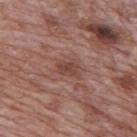Part of a total-body skin-imaging series; this lesion was reviewed on a skin check and was not flagged for biopsy. A male patient, aged approximately 70. Longest diameter approximately 2.5 mm. Automated tile analysis of the lesion measured a lesion color around L≈44 a*≈21 b*≈24 in CIELAB, roughly 8 lightness units darker than nearby skin, and a lesion-to-skin contrast of about 6.5 (normalized; higher = more distinct). It also reported border irregularity of about 2 on a 0–10 scale and a peripheral color-asymmetry measure near 0.5. The lesion is located on the mid back. A close-up tile cropped from a whole-body skin photograph, about 15 mm across. This is a white-light tile.